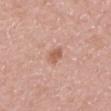Imaged during a routine full-body skin examination; the lesion was not biopsied and no histopathology is available.
The patient is a female in their 30s.
The lesion is on the upper back.
A 15 mm crop from a total-body photograph taken for skin-cancer surveillance.
Imaged with white-light lighting.